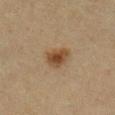Assessment: Captured during whole-body skin photography for melanoma surveillance; the lesion was not biopsied. Image and clinical context: A 15 mm close-up extracted from a 3D total-body photography capture. The lesion-visualizer software estimated an eccentricity of roughly 0.45 and a shape-asymmetry score of about 0.2 (0 = symmetric). And it measured an average lesion color of about L≈39 a*≈15 b*≈29 (CIELAB). A female patient about 55 years old. From the right lower leg.